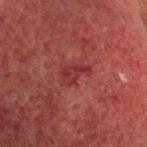Case summary:
– notes · catalogued during a skin exam; not biopsied
– patient · male, aged around 70
– site · the head or neck
– diameter · about 3 mm
– acquisition · total-body-photography crop, ~15 mm field of view